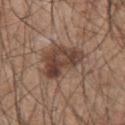- workup · imaged on a skin check; not biopsied
- image · 15 mm crop, total-body photography
- site · the back
- automated metrics · an average lesion color of about L≈44 a*≈17 b*≈25 (CIELAB), about 10 CIELAB-L* units darker than the surrounding skin, and a normalized lesion–skin contrast near 8; a border-irregularity index near 4.5/10, a color-variation rating of about 7/10, and peripheral color asymmetry of about 2.5
- subject · male, aged 43 to 47
- lighting · white-light illumination